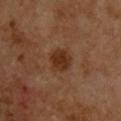Notes:
- workup — imaged on a skin check; not biopsied
- lighting — cross-polarized
- lesion size — about 3 mm
- location — the upper back
- patient — male, about 60 years old
- image — ~15 mm tile from a whole-body skin photo